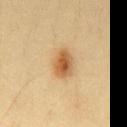<record>
  <biopsy_status>not biopsied; imaged during a skin examination</biopsy_status>
  <automated_metrics>
    <cielab_L>58</cielab_L>
    <cielab_a>20</cielab_a>
    <cielab_b>40</cielab_b>
    <vs_skin_darker_L>13.0</vs_skin_darker_L>
    <vs_skin_contrast_norm>8.5</vs_skin_contrast_norm>
    <nevus_likeness_0_100>100</nevus_likeness_0_100>
    <lesion_detection_confidence_0_100>100</lesion_detection_confidence_0_100>
  </automated_metrics>
  <lesion_size>
    <long_diameter_mm_approx>4.0</long_diameter_mm_approx>
  </lesion_size>
  <image>
    <source>total-body photography crop</source>
    <field_of_view_mm>15</field_of_view_mm>
  </image>
  <site>abdomen</site>
  <lighting>cross-polarized</lighting>
  <patient>
    <sex>male</sex>
    <age_approx>45</age_approx>
  </patient>
</record>